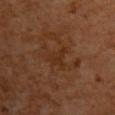notes: imaged on a skin check; not biopsied
automated lesion analysis: a lesion area of about 4.5 mm², an outline eccentricity of about 0.8 (0 = round, 1 = elongated), and a shape-asymmetry score of about 0.5 (0 = symmetric); a nevus-likeness score of about 0/100
patient: male, aged 58–62
location: the upper back
illumination: cross-polarized
lesion size: about 3.5 mm
imaging modality: ~15 mm tile from a whole-body skin photo